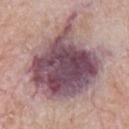notes: imaged on a skin check; not biopsied
lesion diameter: about 10.5 mm
patient: male, aged approximately 65
image source: ~15 mm crop, total-body skin-cancer survey
site: the chest